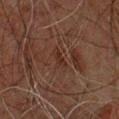Clinical impression:
Captured during whole-body skin photography for melanoma surveillance; the lesion was not biopsied.
Context:
A male subject, aged around 60. The lesion is located on the chest. A 15 mm close-up tile from a total-body photography series done for melanoma screening. Captured under cross-polarized illumination. The total-body-photography lesion software estimated a lesion area of about 3 mm² and an outline eccentricity of about 0.85 (0 = round, 1 = elongated). About 3 mm across.